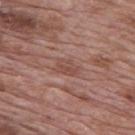notes = total-body-photography surveillance lesion; no biopsy | anatomic site = the mid back | acquisition = ~15 mm tile from a whole-body skin photo | subject = male, approximately 70 years of age.